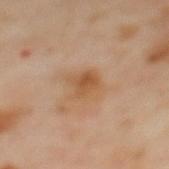Approximately 3 mm at its widest.
Captured under cross-polarized illumination.
The patient is a female aged around 60.
The lesion-visualizer software estimated a lesion area of about 7.5 mm² and an outline eccentricity of about 0.4 (0 = round, 1 = elongated). It also reported a mean CIELAB color near L≈54 a*≈19 b*≈36, about 8 CIELAB-L* units darker than the surrounding skin, and a normalized border contrast of about 7. It also reported a border-irregularity index near 2.5/10 and internal color variation of about 4 on a 0–10 scale.
A region of skin cropped from a whole-body photographic capture, roughly 15 mm wide.
Located on the upper back.